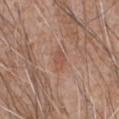Notes:
* notes — total-body-photography surveillance lesion; no biopsy